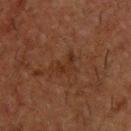{
  "biopsy_status": "not biopsied; imaged during a skin examination",
  "lighting": "cross-polarized",
  "lesion_size": {
    "long_diameter_mm_approx": 3.0
  },
  "patient": {
    "sex": "male",
    "age_approx": 50
  },
  "site": "upper back",
  "image": {
    "source": "total-body photography crop",
    "field_of_view_mm": 15
  }
}